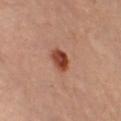This lesion was catalogued during total-body skin photography and was not selected for biopsy. This is a cross-polarized tile. Cropped from a total-body skin-imaging series; the visible field is about 15 mm. The lesion is located on the left leg. The lesion's longest dimension is about 2.5 mm. The lesion-visualizer software estimated a footprint of about 5.5 mm², an outline eccentricity of about 0.5 (0 = round, 1 = elongated), and two-axis asymmetry of about 0.2. It also reported an average lesion color of about L≈38 a*≈24 b*≈28 (CIELAB), about 13 CIELAB-L* units darker than the surrounding skin, and a normalized border contrast of about 11. It also reported border irregularity of about 1.5 on a 0–10 scale, a within-lesion color-variation index near 5/10, and a peripheral color-asymmetry measure near 2. A female subject, about 60 years old.